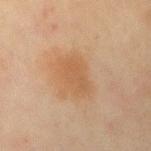notes: catalogued during a skin exam; not biopsied | image: 15 mm crop, total-body photography | subject: female, about 50 years old | site: the chest.